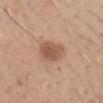Clinical summary:
Longest diameter approximately 3.5 mm. A male subject aged around 30. A 15 mm crop from a total-body photograph taken for skin-cancer surveillance. The lesion is on the right forearm. This is a white-light tile.